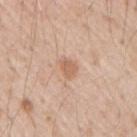biopsy_status: not biopsied; imaged during a skin examination
image:
  source: total-body photography crop
  field_of_view_mm: 15
patient:
  sex: male
  age_approx: 50
site: right upper arm
automated_metrics:
  eccentricity: 0.7
  shape_asymmetry: 0.25
  cielab_L: 62
  cielab_a: 20
  cielab_b: 32
  vs_skin_contrast_norm: 6.5
  nevus_likeness_0_100: 10
  lesion_detection_confidence_0_100: 100
lighting: white-light